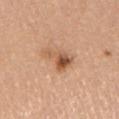Recorded during total-body skin imaging; not selected for excision or biopsy.
This image is a 15 mm lesion crop taken from a total-body photograph.
About 4.5 mm across.
On the right upper arm.
Automated tile analysis of the lesion measured an area of roughly 7.5 mm², an outline eccentricity of about 0.85 (0 = round, 1 = elongated), and a symmetry-axis asymmetry near 0.45. The analysis additionally found an average lesion color of about L≈56 a*≈22 b*≈34 (CIELAB), roughly 12 lightness units darker than nearby skin, and a lesion-to-skin contrast of about 8 (normalized; higher = more distinct).
The tile uses white-light illumination.
A female patient, aged 63–67.